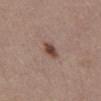workup: no biopsy performed (imaged during a skin exam)
illumination: white-light illumination
diameter: about 3 mm
acquisition: total-body-photography crop, ~15 mm field of view
image-analysis metrics: a footprint of about 4 mm², an eccentricity of roughly 0.8, and a shape-asymmetry score of about 0.15 (0 = symmetric); a mean CIELAB color near L≈45 a*≈19 b*≈24; a border-irregularity rating of about 2/10, internal color variation of about 3 on a 0–10 scale, and peripheral color asymmetry of about 0.5
site: the abdomen
patient: female, roughly 40 years of age